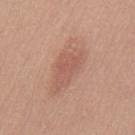Findings:
– notes — no biopsy performed (imaged during a skin exam)
– location — the mid back
– patient — male, roughly 30 years of age
– imaging modality — 15 mm crop, total-body photography